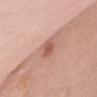| feature | finding |
|---|---|
| workup | catalogued during a skin exam; not biopsied |
| body site | the chest |
| subject | female, aged around 50 |
| image | ~15 mm crop, total-body skin-cancer survey |
| lesion size | about 3 mm |
| tile lighting | white-light illumination |